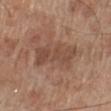Assessment:
Part of a total-body skin-imaging series; this lesion was reviewed on a skin check and was not flagged for biopsy.
Context:
A close-up tile cropped from a whole-body skin photograph, about 15 mm across. The lesion is located on the left lower leg. The subject is a male aged approximately 70.